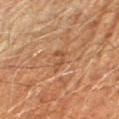Q: Was a biopsy performed?
A: imaged on a skin check; not biopsied
Q: How large is the lesion?
A: about 2.5 mm
Q: Automated lesion metrics?
A: a footprint of about 2.5 mm², an outline eccentricity of about 0.85 (0 = round, 1 = elongated), and a shape-asymmetry score of about 0.55 (0 = symmetric); a lesion color around L≈41 a*≈19 b*≈30 in CIELAB; radial color variation of about 0
Q: Illumination type?
A: cross-polarized
Q: What kind of image is this?
A: ~15 mm crop, total-body skin-cancer survey
Q: What is the anatomic site?
A: the right forearm
Q: Who is the patient?
A: male, aged approximately 70